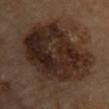Part of a total-body skin-imaging series; this lesion was reviewed on a skin check and was not flagged for biopsy. About 11.5 mm across. The lesion is on the front of the torso. Captured under cross-polarized illumination. The lesion-visualizer software estimated a lesion area of about 65 mm², a shape eccentricity near 0.7, and two-axis asymmetry of about 0.25. The analysis additionally found a border-irregularity rating of about 3.5/10, a within-lesion color-variation index near 7/10, and radial color variation of about 2.5. It also reported an automated nevus-likeness rating near 20 out of 100 and lesion-presence confidence of about 100/100. A roughly 15 mm field-of-view crop from a total-body skin photograph.